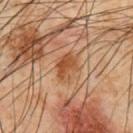No biopsy was performed on this lesion — it was imaged during a full skin examination and was not determined to be concerning. A lesion tile, about 15 mm wide, cut from a 3D total-body photograph. Located on the chest. The subject is a male in their mid- to late 60s.